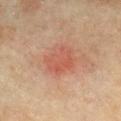Captured during whole-body skin photography for melanoma surveillance; the lesion was not biopsied. A 15 mm crop from a total-body photograph taken for skin-cancer surveillance. Automated image analysis of the tile measured a footprint of about 11 mm², a shape eccentricity near 0.55, and a symmetry-axis asymmetry near 0.2. The analysis additionally found internal color variation of about 3 on a 0–10 scale and radial color variation of about 1. The analysis additionally found a classifier nevus-likeness of about 0/100. Captured under cross-polarized illumination. The lesion is on the chest. The lesion's longest dimension is about 4 mm. The subject is a female roughly 70 years of age.